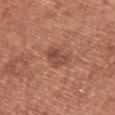Findings:
- follow-up: no biopsy performed (imaged during a skin exam)
- location: the chest
- image source: total-body-photography crop, ~15 mm field of view
- lesion size: ~3.5 mm (longest diameter)
- patient: female, about 65 years old